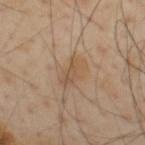{
  "biopsy_status": "not biopsied; imaged during a skin examination",
  "image": {
    "source": "total-body photography crop",
    "field_of_view_mm": 15
  },
  "site": "upper back",
  "lesion_size": {
    "long_diameter_mm_approx": 3.5
  },
  "patient": {
    "sex": "male",
    "age_approx": 55
  }
}